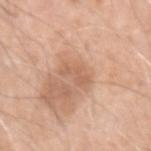About 3.5 mm across. A lesion tile, about 15 mm wide, cut from a 3D total-body photograph. The lesion is located on the arm. Imaged with white-light lighting. A male subject aged approximately 60.